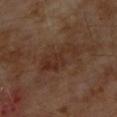Notes:
* follow-up — catalogued during a skin exam; not biopsied
* subject — male, about 70 years old
* image source — ~15 mm crop, total-body skin-cancer survey
* anatomic site — the upper back